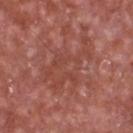Findings:
- notes — catalogued during a skin exam; not biopsied
- image source — ~15 mm crop, total-body skin-cancer survey
- subject — male, approximately 65 years of age
- automated lesion analysis — an area of roughly 21 mm², an eccentricity of roughly 0.85, and a symmetry-axis asymmetry near 0.5; roughly 6 lightness units darker than nearby skin and a normalized border contrast of about 5
- location — the front of the torso
- tile lighting — white-light illumination